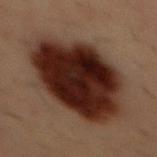This lesion was catalogued during total-body skin photography and was not selected for biopsy. Cropped from a whole-body photographic skin survey; the tile spans about 15 mm. From the front of the torso. The lesion's longest dimension is about 10 mm. A male subject approximately 55 years of age.